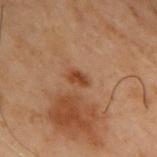Case summary:
* follow-up · catalogued during a skin exam; not biopsied
* size · ≈2.5 mm
* lighting · cross-polarized
* location · the upper back
* image · ~15 mm crop, total-body skin-cancer survey
* automated metrics · a footprint of about 3 mm², an eccentricity of roughly 0.8, and two-axis asymmetry of about 0.3; a mean CIELAB color near L≈35 a*≈20 b*≈29, about 8 CIELAB-L* units darker than the surrounding skin, and a normalized border contrast of about 8; border irregularity of about 3 on a 0–10 scale, a color-variation rating of about 2/10, and radial color variation of about 1; a nevus-likeness score of about 65/100 and a detector confidence of about 100 out of 100 that the crop contains a lesion
* patient · male, aged around 55